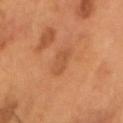<record>
<biopsy_status>not biopsied; imaged during a skin examination</biopsy_status>
<site>head or neck</site>
<patient>
  <sex>male</sex>
  <age_approx>55</age_approx>
</patient>
<image>
  <source>total-body photography crop</source>
  <field_of_view_mm>15</field_of_view_mm>
</image>
<lesion_size>
  <long_diameter_mm_approx>2.5</long_diameter_mm_approx>
</lesion_size>
</record>